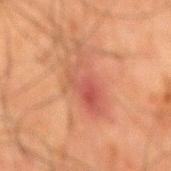Clinical impression: The lesion was tiled from a total-body skin photograph and was not biopsied. Background: The lesion is located on the mid back. A male subject aged around 65. Imaged with cross-polarized lighting. A 15 mm close-up extracted from a 3D total-body photography capture.